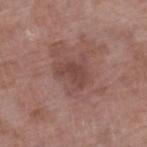  biopsy_status: not biopsied; imaged during a skin examination
  site: right lower leg
  lighting: white-light
  patient:
    sex: female
    age_approx: 70
  lesion_size:
    long_diameter_mm_approx: 3.5
  automated_metrics:
    border_irregularity_0_10: 3.5
    color_variation_0_10: 1.5
    peripheral_color_asymmetry: 0.5
  image:
    source: total-body photography crop
    field_of_view_mm: 15